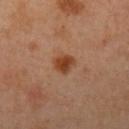Assessment: The lesion was tiled from a total-body skin photograph and was not biopsied. Acquisition and patient details: A 15 mm crop from a total-body photograph taken for skin-cancer surveillance. This is a cross-polarized tile. The subject is a female approximately 40 years of age. The lesion is located on the left upper arm.